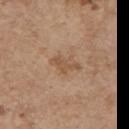Q: Was a biopsy performed?
A: catalogued during a skin exam; not biopsied
Q: What is the anatomic site?
A: the right upper arm
Q: What did automated image analysis measure?
A: a lesion area of about 5 mm², an outline eccentricity of about 0.8 (0 = round, 1 = elongated), and two-axis asymmetry of about 0.4; a detector confidence of about 100 out of 100 that the crop contains a lesion
Q: Lesion size?
A: about 3.5 mm
Q: What lighting was used for the tile?
A: white-light
Q: What are the patient's age and sex?
A: female, roughly 65 years of age
Q: What is the imaging modality?
A: ~15 mm crop, total-body skin-cancer survey